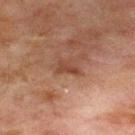Part of a total-body skin-imaging series; this lesion was reviewed on a skin check and was not flagged for biopsy.
On the chest.
An algorithmic analysis of the crop reported an area of roughly 3.5 mm² and a shape eccentricity near 0.85. The software also gave a lesion color around L≈35 a*≈19 b*≈25 in CIELAB, about 7 CIELAB-L* units darker than the surrounding skin, and a normalized border contrast of about 6.5.
This image is a 15 mm lesion crop taken from a total-body photograph.
The tile uses cross-polarized illumination.
A male patient, in their mid-60s.
The recorded lesion diameter is about 3 mm.